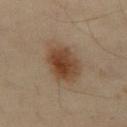| feature | finding |
|---|---|
| follow-up | total-body-photography surveillance lesion; no biopsy |
| image source | ~15 mm crop, total-body skin-cancer survey |
| TBP lesion metrics | a mean CIELAB color near L≈40 a*≈16 b*≈28, roughly 11 lightness units darker than nearby skin, and a normalized border contrast of about 9.5; a detector confidence of about 100 out of 100 that the crop contains a lesion |
| site | the mid back |
| subject | male, in their mid- to late 60s |